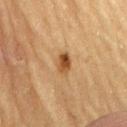biopsy_status: not biopsied; imaged during a skin examination
image:
  source: total-body photography crop
  field_of_view_mm: 15
patient:
  sex: male
  age_approx: 85+
site: lower back